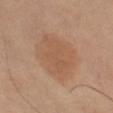Located on the right leg. The subject is a female in their 60s. Longest diameter approximately 6.5 mm. A 15 mm crop from a total-body photograph taken for skin-cancer surveillance.